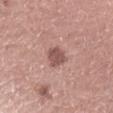image: ~15 mm crop, total-body skin-cancer survey | patient: female, about 50 years old | lighting: white-light illumination | automated metrics: a footprint of about 5 mm²; an average lesion color of about L≈52 a*≈22 b*≈23 (CIELAB), a lesion–skin lightness drop of about 12, and a lesion-to-skin contrast of about 8 (normalized; higher = more distinct); a color-variation rating of about 1.5/10 and a peripheral color-asymmetry measure near 0.5; a classifier nevus-likeness of about 35/100 and a lesion-detection confidence of about 100/100 | site: the arm | diameter: ≈2.5 mm.